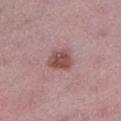Impression: The lesion was photographed on a routine skin check and not biopsied; there is no pathology result. Clinical summary: Located on the leg. The lesion-visualizer software estimated an area of roughly 7.5 mm² and an eccentricity of roughly 0.6. It also reported a mean CIELAB color near L≈50 a*≈22 b*≈21, a lesion–skin lightness drop of about 12, and a normalized lesion–skin contrast near 8.5. It also reported border irregularity of about 2.5 on a 0–10 scale and peripheral color asymmetry of about 1. A female patient about 45 years old. A 15 mm crop from a total-body photograph taken for skin-cancer surveillance. The tile uses white-light illumination.